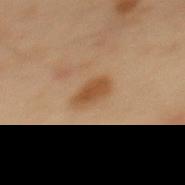The lesion was tiled from a total-body skin photograph and was not biopsied.